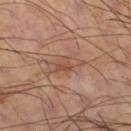Recorded during total-body skin imaging; not selected for excision or biopsy. The lesion-visualizer software estimated a lesion area of about 5.5 mm², an eccentricity of roughly 0.9, and a shape-asymmetry score of about 0.35 (0 = symmetric). The lesion's longest dimension is about 4.5 mm. Captured under cross-polarized illumination. A 15 mm close-up tile from a total-body photography series done for melanoma screening. A male subject, roughly 60 years of age. On the right thigh.